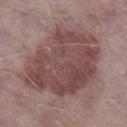Q: Was this lesion biopsied?
A: imaged on a skin check; not biopsied
Q: Patient demographics?
A: male, aged approximately 75
Q: What did automated image analysis measure?
A: an area of roughly 55 mm², a shape eccentricity near 0.65, and a symmetry-axis asymmetry near 0.2
Q: What is the imaging modality?
A: total-body-photography crop, ~15 mm field of view
Q: How large is the lesion?
A: about 9.5 mm
Q: Lesion location?
A: the right lower leg